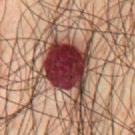No biopsy was performed on this lesion — it was imaged during a full skin examination and was not determined to be concerning. From the chest. A male patient, roughly 45 years of age. Captured under cross-polarized illumination. A 15 mm close-up extracted from a 3D total-body photography capture.